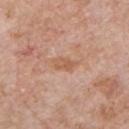Part of a total-body skin-imaging series; this lesion was reviewed on a skin check and was not flagged for biopsy. Cropped from a total-body skin-imaging series; the visible field is about 15 mm. A male patient about 60 years old. The lesion is located on the chest.